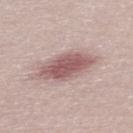{
  "biopsy_status": "not biopsied; imaged during a skin examination",
  "patient": {
    "sex": "male",
    "age_approx": 30
  },
  "image": {
    "source": "total-body photography crop",
    "field_of_view_mm": 15
  }
}